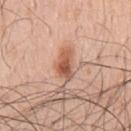This lesion was catalogued during total-body skin photography and was not selected for biopsy. Imaged with white-light lighting. A 15 mm close-up tile from a total-body photography series done for melanoma screening. The patient is a male in their mid-50s. The lesion is on the chest.